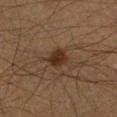Clinical summary:
A male subject, approximately 40 years of age. A close-up tile cropped from a whole-body skin photograph, about 15 mm across. Captured under cross-polarized illumination. Located on the left lower leg. Automated image analysis of the tile measured a classifier nevus-likeness of about 90/100.